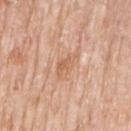| key | value |
|---|---|
| biopsy status | total-body-photography surveillance lesion; no biopsy |
| subject | female, aged approximately 85 or older |
| diameter | about 3.5 mm |
| body site | the right upper arm |
| tile lighting | white-light |
| automated metrics | a border-irregularity rating of about 3.5/10 and internal color variation of about 2.5 on a 0–10 scale; a lesion-detection confidence of about 100/100 |
| image source | total-body-photography crop, ~15 mm field of view |